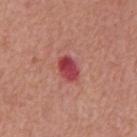image source = ~15 mm tile from a whole-body skin photo; size = ≈3.5 mm; patient = male, aged 63–67; body site = the mid back.